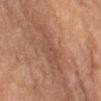biopsy_status: not biopsied; imaged during a skin examination
automated_metrics:
  area_mm2_approx: 9.5
  shape_asymmetry: 0.35
  lesion_detection_confidence_0_100: 70
site: left lower leg
lighting: cross-polarized
image:
  source: total-body photography crop
  field_of_view_mm: 15
patient:
  sex: female
  age_approx: 55
lesion_size:
  long_diameter_mm_approx: 6.5